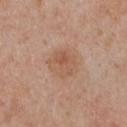workup = catalogued during a skin exam; not biopsied
diameter = about 3.5 mm
subject = female, aged around 55
location = the front of the torso
acquisition = ~15 mm tile from a whole-body skin photo
tile lighting = white-light illumination